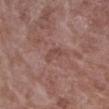{"biopsy_status": "not biopsied; imaged during a skin examination"}